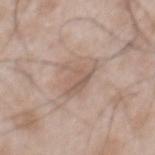A male subject in their 50s.
The lesion is on the mid back.
Cropped from a total-body skin-imaging series; the visible field is about 15 mm.